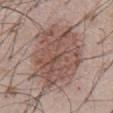This lesion was catalogued during total-body skin photography and was not selected for biopsy.
A 15 mm crop from a total-body photograph taken for skin-cancer surveillance.
From the abdomen.
A male subject aged approximately 55.